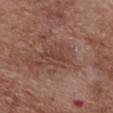* notes — no biopsy performed (imaged during a skin exam)
* image — 15 mm crop, total-body photography
* subject — female, aged around 80
* site — the chest